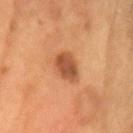notes = catalogued during a skin exam; not biopsied | body site = the head or neck | lighting = cross-polarized illumination | subject = male, roughly 55 years of age | automated metrics = an area of roughly 7 mm² and two-axis asymmetry of about 0.2; a lesion color around L≈47 a*≈25 b*≈35 in CIELAB and a normalized border contrast of about 8.5; border irregularity of about 2 on a 0–10 scale and a peripheral color-asymmetry measure near 1 | lesion size = ~3 mm (longest diameter) | acquisition = ~15 mm crop, total-body skin-cancer survey.